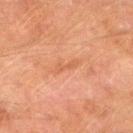biopsy_status: not biopsied; imaged during a skin examination
image:
  source: total-body photography crop
  field_of_view_mm: 15
site: right thigh
patient:
  sex: male
  age_approx: 75